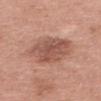Q: Was a biopsy performed?
A: imaged on a skin check; not biopsied